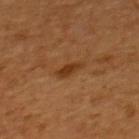Clinical impression: No biopsy was performed on this lesion — it was imaged during a full skin examination and was not determined to be concerning. Clinical summary: Captured under cross-polarized illumination. A male patient, in their mid-40s. A roughly 15 mm field-of-view crop from a total-body skin photograph. On the upper back. An algorithmic analysis of the crop reported a lesion area of about 3.5 mm², an outline eccentricity of about 0.9 (0 = round, 1 = elongated), and a symmetry-axis asymmetry near 0.25. It also reported a lesion color around L≈33 a*≈22 b*≈34 in CIELAB and roughly 9 lightness units darker than nearby skin. The analysis additionally found a nevus-likeness score of about 60/100.